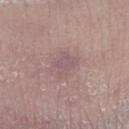Findings:
* notes · total-body-photography surveillance lesion; no biopsy
* image · 15 mm crop, total-body photography
* automated lesion analysis · a footprint of about 7.5 mm², an outline eccentricity of about 0.6 (0 = round, 1 = elongated), and a symmetry-axis asymmetry near 0.2; border irregularity of about 2 on a 0–10 scale, a within-lesion color-variation index near 1.5/10, and a peripheral color-asymmetry measure near 0.5
* subject · male, aged approximately 65
* site · the leg
* lesion size · about 3.5 mm